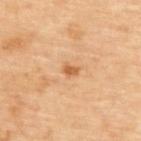Part of a total-body skin-imaging series; this lesion was reviewed on a skin check and was not flagged for biopsy.